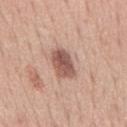No biopsy was performed on this lesion — it was imaged during a full skin examination and was not determined to be concerning. This is a white-light tile. A male subject in their 50s. The lesion's longest dimension is about 4 mm. A roughly 15 mm field-of-view crop from a total-body skin photograph. The total-body-photography lesion software estimated an area of roughly 8.5 mm², an eccentricity of roughly 0.85, and a shape-asymmetry score of about 0.2 (0 = symmetric). The analysis additionally found a mean CIELAB color near L≈54 a*≈21 b*≈25. It also reported a border-irregularity index near 2.5/10, a color-variation rating of about 5/10, and radial color variation of about 2. On the mid back.